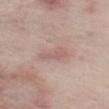Imaged during a routine full-body skin examination; the lesion was not biopsied and no histopathology is available. A lesion tile, about 15 mm wide, cut from a 3D total-body photograph. On the left thigh. Captured under white-light illumination. A male patient, aged around 65.